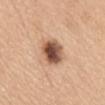Part of a total-body skin-imaging series; this lesion was reviewed on a skin check and was not flagged for biopsy.
A 15 mm close-up extracted from a 3D total-body photography capture.
The total-body-photography lesion software estimated a mean CIELAB color near L≈53 a*≈21 b*≈31, roughly 19 lightness units darker than nearby skin, and a lesion-to-skin contrast of about 12 (normalized; higher = more distinct). It also reported border irregularity of about 1.5 on a 0–10 scale, internal color variation of about 8 on a 0–10 scale, and radial color variation of about 2.5.
The patient is a female about 65 years old.
Captured under white-light illumination.
On the mid back.